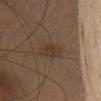biopsy status — no biopsy performed (imaged during a skin exam) | illumination — cross-polarized illumination | automated lesion analysis — an area of roughly 5 mm² and a shape-asymmetry score of about 0.15 (0 = symmetric); an automated nevus-likeness rating near 5 out of 100 and a detector confidence of about 100 out of 100 that the crop contains a lesion | acquisition — ~15 mm crop, total-body skin-cancer survey | diameter — ~3 mm (longest diameter) | site — the head or neck | patient — female, about 40 years old.